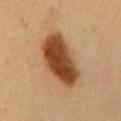{
  "biopsy_status": "not biopsied; imaged during a skin examination",
  "lighting": "cross-polarized",
  "lesion_size": {
    "long_diameter_mm_approx": 6.5
  },
  "site": "mid back",
  "image": {
    "source": "total-body photography crop",
    "field_of_view_mm": 15
  },
  "patient": {
    "sex": "male",
    "age_approx": 50
  },
  "automated_metrics": {
    "area_mm2_approx": 23.0,
    "eccentricity": 0.75,
    "border_irregularity_0_10": 2.0,
    "color_variation_0_10": 6.5,
    "peripheral_color_asymmetry": 2.0,
    "lesion_detection_confidence_0_100": 100
  }
}